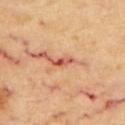Imaged during a routine full-body skin examination; the lesion was not biopsied and no histopathology is available.
This is a cross-polarized tile.
A male subject, aged approximately 70.
From the back.
The lesion-visualizer software estimated a lesion color around L≈54 a*≈32 b*≈32 in CIELAB, roughly 14 lightness units darker than nearby skin, and a lesion-to-skin contrast of about 9.5 (normalized; higher = more distinct). And it measured border irregularity of about 3 on a 0–10 scale and a within-lesion color-variation index near 0/10. The software also gave an automated nevus-likeness rating near 0 out of 100 and a lesion-detection confidence of about 95/100.
Cropped from a total-body skin-imaging series; the visible field is about 15 mm.